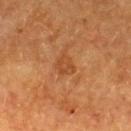Clinical impression: Captured during whole-body skin photography for melanoma surveillance; the lesion was not biopsied. Acquisition and patient details: A female subject, in their mid-50s. Located on the upper back. A 15 mm close-up extracted from a 3D total-body photography capture. Approximately 3 mm at its widest.